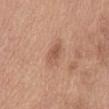This is a white-light tile. A female patient roughly 55 years of age. A region of skin cropped from a whole-body photographic capture, roughly 15 mm wide. Longest diameter approximately 3 mm. Automated image analysis of the tile measured a footprint of about 5 mm², a shape eccentricity near 0.75, and two-axis asymmetry of about 0.15. And it measured a lesion color around L≈55 a*≈22 b*≈31 in CIELAB, about 9 CIELAB-L* units darker than the surrounding skin, and a normalized border contrast of about 6. And it measured a within-lesion color-variation index near 3.5/10 and peripheral color asymmetry of about 1.5. The lesion is on the front of the torso.